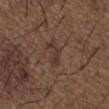Impression:
Imaged during a routine full-body skin examination; the lesion was not biopsied and no histopathology is available.
Background:
About 2.5 mm across. Automated image analysis of the tile measured an automated nevus-likeness rating near 0 out of 100 and a lesion-detection confidence of about 95/100. A male subject, in their 50s. Located on the upper back. Captured under white-light illumination. Cropped from a whole-body photographic skin survey; the tile spans about 15 mm.